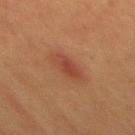biopsy status: total-body-photography surveillance lesion; no biopsy
automated lesion analysis: a border-irregularity rating of about 2/10 and a within-lesion color-variation index near 3/10
lighting: cross-polarized illumination
location: the back
subject: male, aged around 40
lesion size: about 4 mm
acquisition: ~15 mm crop, total-body skin-cancer survey